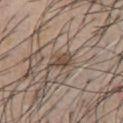  biopsy_status: not biopsied; imaged during a skin examination
  image:
    source: total-body photography crop
    field_of_view_mm: 15
  site: chest
  lesion_size:
    long_diameter_mm_approx: 3.5
  lighting: white-light
  patient:
    sex: male
    age_approx: 55
  automated_metrics:
    lesion_detection_confidence_0_100: 60